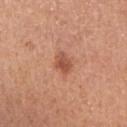Acquisition and patient details: A close-up tile cropped from a whole-body skin photograph, about 15 mm across. On the right upper arm. The subject is a female aged approximately 50. Approximately 3 mm at its widest. The tile uses white-light illumination. The lesion-visualizer software estimated an area of roughly 4.5 mm², a shape eccentricity near 0.8, and two-axis asymmetry of about 0.2. The software also gave a border-irregularity index near 2/10, a within-lesion color-variation index near 3.5/10, and a peripheral color-asymmetry measure near 1. The analysis additionally found a nevus-likeness score of about 75/100.